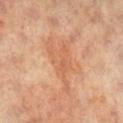Recorded during total-body skin imaging; not selected for excision or biopsy.
An algorithmic analysis of the crop reported a mean CIELAB color near L≈60 a*≈25 b*≈35. The software also gave border irregularity of about 8.5 on a 0–10 scale, internal color variation of about 3 on a 0–10 scale, and a peripheral color-asymmetry measure near 1. The analysis additionally found an automated nevus-likeness rating near 0 out of 100 and lesion-presence confidence of about 100/100.
A 15 mm close-up extracted from a 3D total-body photography capture.
The tile uses cross-polarized illumination.
Located on the left leg.
A female patient, in their mid- to late 60s.